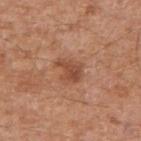Measured at roughly 3 mm in maximum diameter. Captured under white-light illumination. This image is a 15 mm lesion crop taken from a total-body photograph. The lesion is on the right upper arm. A male patient, approximately 60 years of age.